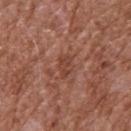A 15 mm crop from a total-body photograph taken for skin-cancer surveillance. The lesion is located on the back. The patient is a male about 75 years old. Captured under white-light illumination.